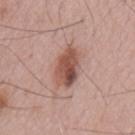patient: male, aged around 55 | imaging modality: ~15 mm crop, total-body skin-cancer survey.